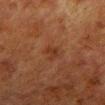  biopsy_status: not biopsied; imaged during a skin examination
  lesion_size:
    long_diameter_mm_approx: 2.5
  image:
    source: total-body photography crop
    field_of_view_mm: 15
  site: left lower leg
  patient:
    sex: male
    age_approx: 80
  lighting: cross-polarized
  automated_metrics:
    area_mm2_approx: 3.0
    eccentricity: 0.85
    shape_asymmetry: 0.3
    vs_skin_darker_L: 5.0
    border_irregularity_0_10: 3.5
    color_variation_0_10: 1.0
    peripheral_color_asymmetry: 0.0
    nevus_likeness_0_100: 10
    lesion_detection_confidence_0_100: 100